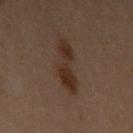Imaged during a routine full-body skin examination; the lesion was not biopsied and no histopathology is available. The recorded lesion diameter is about 5 mm. Cropped from a total-body skin-imaging series; the visible field is about 15 mm. Located on the left upper arm. The lesion-visualizer software estimated an eccentricity of roughly 0.9 and a shape-asymmetry score of about 0.35 (0 = symmetric). The analysis additionally found a mean CIELAB color near L≈22 a*≈13 b*≈20 and a normalized border contrast of about 8.5. The software also gave border irregularity of about 5 on a 0–10 scale, a color-variation rating of about 2.5/10, and peripheral color asymmetry of about 1. The tile uses cross-polarized illumination. A female patient aged 28–32.